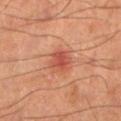{"biopsy_status": "not biopsied; imaged during a skin examination", "site": "right lower leg", "image": {"source": "total-body photography crop", "field_of_view_mm": 15}, "patient": {"sex": "male", "age_approx": 65}, "lesion_size": {"long_diameter_mm_approx": 2.5}, "automated_metrics": {"border_irregularity_0_10": 2.0, "color_variation_0_10": 3.0}}